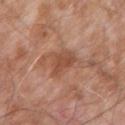The lesion was photographed on a routine skin check and not biopsied; there is no pathology result.
The lesion's longest dimension is about 4 mm.
This is a white-light tile.
Cropped from a total-body skin-imaging series; the visible field is about 15 mm.
The lesion-visualizer software estimated about 9 CIELAB-L* units darker than the surrounding skin and a normalized border contrast of about 7. It also reported a color-variation rating of about 2/10 and radial color variation of about 0.5.
The lesion is located on the right upper arm.
A male patient aged 73 to 77.